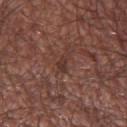biopsy_status: not biopsied; imaged during a skin examination
image:
  source: total-body photography crop
  field_of_view_mm: 15
site: left lower leg
lesion_size:
  long_diameter_mm_approx: 3.0
patient:
  sex: male
  age_approx: 60